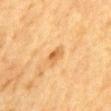Captured during whole-body skin photography for melanoma surveillance; the lesion was not biopsied.
Located on the abdomen.
A roughly 15 mm field-of-view crop from a total-body skin photograph.
The subject is a male about 85 years old or older.